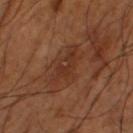Q: Is there a histopathology result?
A: total-body-photography surveillance lesion; no biopsy
Q: How was this image acquired?
A: total-body-photography crop, ~15 mm field of view
Q: What is the anatomic site?
A: the leg
Q: Illumination type?
A: cross-polarized
Q: Lesion size?
A: about 5 mm
Q: What are the patient's age and sex?
A: male, in their mid-60s